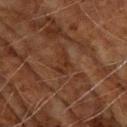Impression:
Captured during whole-body skin photography for melanoma surveillance; the lesion was not biopsied.
Image and clinical context:
The tile uses cross-polarized illumination. Located on the right upper arm. The subject is a male in their 70s. A close-up tile cropped from a whole-body skin photograph, about 15 mm across.